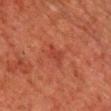Part of a total-body skin-imaging series; this lesion was reviewed on a skin check and was not flagged for biopsy.
Located on the chest.
A region of skin cropped from a whole-body photographic capture, roughly 15 mm wide.
A male subject, roughly 60 years of age.
The tile uses cross-polarized illumination.
The recorded lesion diameter is about 3 mm.